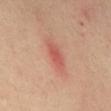Notes:
– workup — imaged on a skin check; not biopsied
– image — 15 mm crop, total-body photography
– patient — female, approximately 40 years of age
– anatomic site — the mid back